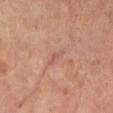<lesion>
<biopsy_status>not biopsied; imaged during a skin examination</biopsy_status>
<image>
  <source>total-body photography crop</source>
  <field_of_view_mm>15</field_of_view_mm>
</image>
<patient>
  <sex>female</sex>
  <age_approx>65</age_approx>
</patient>
<lesion_size>
  <long_diameter_mm_approx>2.5</long_diameter_mm_approx>
</lesion_size>
<site>right leg</site>
<lighting>cross-polarized</lighting>
</lesion>